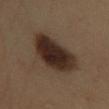Recorded during total-body skin imaging; not selected for excision or biopsy. Captured under cross-polarized illumination. About 6.5 mm across. The subject is a female approximately 50 years of age. The total-body-photography lesion software estimated an average lesion color of about L≈29 a*≈14 b*≈22 (CIELAB) and a lesion-to-skin contrast of about 13 (normalized; higher = more distinct). The software also gave a classifier nevus-likeness of about 95/100 and a detector confidence of about 100 out of 100 that the crop contains a lesion. The lesion is on the left lower leg. A 15 mm close-up tile from a total-body photography series done for melanoma screening.